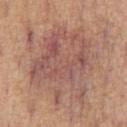{"biopsy_status": "not biopsied; imaged during a skin examination", "lesion_size": {"long_diameter_mm_approx": 10.5}, "site": "chest", "lighting": "white-light", "patient": {"sex": "male", "age_approx": 60}, "image": {"source": "total-body photography crop", "field_of_view_mm": 15}}